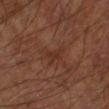location: the arm | illumination: cross-polarized illumination | acquisition: total-body-photography crop, ~15 mm field of view | patient: male, approximately 70 years of age.